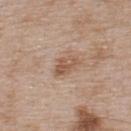The lesion was photographed on a routine skin check and not biopsied; there is no pathology result. The tile uses white-light illumination. Approximately 3 mm at its widest. On the upper back. The subject is a male approximately 55 years of age. A lesion tile, about 15 mm wide, cut from a 3D total-body photograph.